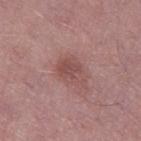biopsy_status: not biopsied; imaged during a skin examination
lighting: white-light
patient:
  sex: male
  age_approx: 55
site: left thigh
image:
  source: total-body photography crop
  field_of_view_mm: 15
automated_metrics:
  shape_asymmetry: 0.3
  cielab_L: 49
  cielab_a: 23
  cielab_b: 21
  vs_skin_darker_L: 8.0
  vs_skin_contrast_norm: 6.0
  border_irregularity_0_10: 3.0
  color_variation_0_10: 2.5
  nevus_likeness_0_100: 5
  lesion_detection_confidence_0_100: 100